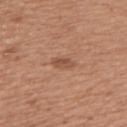| feature | finding |
|---|---|
| biopsy status | no biopsy performed (imaged during a skin exam) |
| subject | female, aged 53–57 |
| size | about 3 mm |
| location | the back |
| acquisition | ~15 mm tile from a whole-body skin photo |
| automated lesion analysis | a border-irregularity rating of about 2/10 and internal color variation of about 1.5 on a 0–10 scale; lesion-presence confidence of about 100/100 |